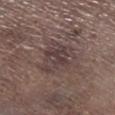The lesion was tiled from a total-body skin photograph and was not biopsied. Longest diameter approximately 4.5 mm. The subject is a male about 70 years old. A 15 mm crop from a total-body photograph taken for skin-cancer surveillance. From the right lower leg.